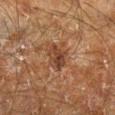workup: imaged on a skin check; not biopsied
subject: male, roughly 60 years of age
automated lesion analysis: a lesion color around L≈31 a*≈17 b*≈25 in CIELAB, a lesion–skin lightness drop of about 8, and a normalized border contrast of about 7.5; a nevus-likeness score of about 0/100 and lesion-presence confidence of about 100/100
location: the right lower leg
diameter: ≈3 mm
image: total-body-photography crop, ~15 mm field of view